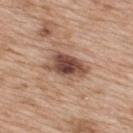notes: catalogued during a skin exam; not biopsied
site: the upper back
acquisition: ~15 mm tile from a whole-body skin photo
tile lighting: white-light illumination
size: ≈5 mm
subject: female, in their 40s